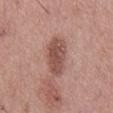Assessment:
This lesion was catalogued during total-body skin photography and was not selected for biopsy.
Background:
An algorithmic analysis of the crop reported an average lesion color of about L≈50 a*≈23 b*≈25 (CIELAB), about 12 CIELAB-L* units darker than the surrounding skin, and a lesion-to-skin contrast of about 8.5 (normalized; higher = more distinct). The software also gave a classifier nevus-likeness of about 70/100 and a detector confidence of about 100 out of 100 that the crop contains a lesion. This is a white-light tile. Longest diameter approximately 6 mm. A roughly 15 mm field-of-view crop from a total-body skin photograph. A male subject, in their mid-50s. The lesion is on the mid back.